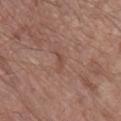* notes · no biopsy performed (imaged during a skin exam)
* automated metrics · an area of roughly 2.5 mm² and two-axis asymmetry of about 0.45
* site · the right lower leg
* lighting · white-light
* acquisition · 15 mm crop, total-body photography
* subject · male, aged approximately 80